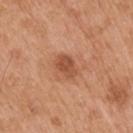The lesion was photographed on a routine skin check and not biopsied; there is no pathology result.
A male subject, aged approximately 55.
A 15 mm close-up extracted from a 3D total-body photography capture.
The lesion is on the right upper arm.